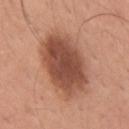follow-up = total-body-photography surveillance lesion; no biopsy
acquisition = 15 mm crop, total-body photography
patient = male, aged 28–32
TBP lesion metrics = an area of roughly 30 mm² and two-axis asymmetry of about 0.1; a mean CIELAB color near L≈49 a*≈24 b*≈30, roughly 15 lightness units darker than nearby skin, and a normalized border contrast of about 10
anatomic site = the right upper arm
illumination = white-light illumination
size = ~7 mm (longest diameter)